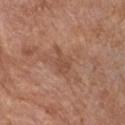workup: no biopsy performed (imaged during a skin exam); image: 15 mm crop, total-body photography; body site: the chest; subject: female, in their mid-70s.